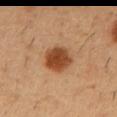<case>
<biopsy_status>not biopsied; imaged during a skin examination</biopsy_status>
<lesion_size>
  <long_diameter_mm_approx>4.0</long_diameter_mm_approx>
</lesion_size>
<site>abdomen</site>
<image>
  <source>total-body photography crop</source>
  <field_of_view_mm>15</field_of_view_mm>
</image>
<automated_metrics>
  <area_mm2_approx>11.0</area_mm2_approx>
  <eccentricity>0.65</eccentricity>
  <shape_asymmetry>0.1</shape_asymmetry>
</automated_metrics>
<lighting>cross-polarized</lighting>
<patient>
  <sex>male</sex>
  <age_approx>50</age_approx>
</patient>
</case>